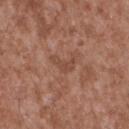follow-up — imaged on a skin check; not biopsied
site — the upper back
acquisition — ~15 mm crop, total-body skin-cancer survey
subject — male, about 45 years old
lesion diameter — about 3 mm